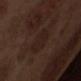Captured during whole-body skin photography for melanoma surveillance; the lesion was not biopsied.
The subject is a male roughly 70 years of age.
The lesion is located on the abdomen.
About 4 mm across.
A lesion tile, about 15 mm wide, cut from a 3D total-body photograph.
The total-body-photography lesion software estimated a peripheral color-asymmetry measure near 0.5.